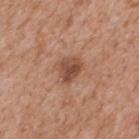<tbp_lesion>
  <biopsy_status>not biopsied; imaged during a skin examination</biopsy_status>
  <image>
    <source>total-body photography crop</source>
    <field_of_view_mm>15</field_of_view_mm>
  </image>
  <lesion_size>
    <long_diameter_mm_approx>3.0</long_diameter_mm_approx>
  </lesion_size>
  <patient>
    <sex>male</sex>
    <age_approx>65</age_approx>
  </patient>
  <site>mid back</site>
</tbp_lesion>